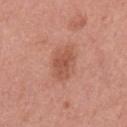follow-up = no biopsy performed (imaged during a skin exam) | automated metrics = a footprint of about 10 mm², a shape eccentricity near 0.7, and two-axis asymmetry of about 0.2; a mean CIELAB color near L≈54 a*≈25 b*≈31 | lighting = white-light illumination | patient = female, approximately 50 years of age | image source = ~15 mm crop, total-body skin-cancer survey | site = the left upper arm.